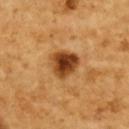Imaged during a routine full-body skin examination; the lesion was not biopsied and no histopathology is available. The total-body-photography lesion software estimated a footprint of about 9 mm² and a shape-asymmetry score of about 0.25 (0 = symmetric). The analysis additionally found a lesion–skin lightness drop of about 18 and a normalized border contrast of about 12.5. It also reported a border-irregularity index near 2/10, a color-variation rating of about 6.5/10, and peripheral color asymmetry of about 2. The analysis additionally found a classifier nevus-likeness of about 95/100 and a lesion-detection confidence of about 100/100. The lesion's longest dimension is about 3.5 mm. A region of skin cropped from a whole-body photographic capture, roughly 15 mm wide. This is a cross-polarized tile. A female subject aged 53 to 57. From the upper back.